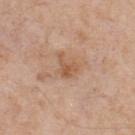This lesion was catalogued during total-body skin photography and was not selected for biopsy. Longest diameter approximately 2.5 mm. Automated image analysis of the tile measured an automated nevus-likeness rating near 0 out of 100. Located on the upper back. Cropped from a whole-body photographic skin survey; the tile spans about 15 mm. Captured under white-light illumination. A male patient roughly 55 years of age.